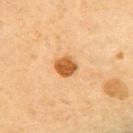Clinical impression:
The lesion was photographed on a routine skin check and not biopsied; there is no pathology result.
Background:
On the back. A 15 mm close-up tile from a total-body photography series done for melanoma screening. Measured at roughly 2.5 mm in maximum diameter. A female patient about 65 years old. Imaged with cross-polarized lighting.